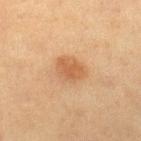The lesion was tiled from a total-body skin photograph and was not biopsied. On the right lower leg. The patient is a female aged 48 to 52. The total-body-photography lesion software estimated a shape-asymmetry score of about 0.25 (0 = symmetric). About 3.5 mm across. This image is a 15 mm lesion crop taken from a total-body photograph. Captured under cross-polarized illumination.